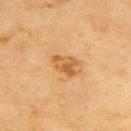{
  "biopsy_status": "not biopsied; imaged during a skin examination"
}